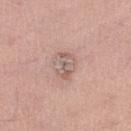Recorded during total-body skin imaging; not selected for excision or biopsy.
A male patient aged approximately 55.
A 15 mm crop from a total-body photograph taken for skin-cancer surveillance.
From the left lower leg.